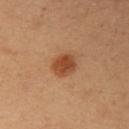Q: Was a biopsy performed?
A: total-body-photography surveillance lesion; no biopsy
Q: What kind of image is this?
A: ~15 mm crop, total-body skin-cancer survey
Q: What is the anatomic site?
A: the right upper arm
Q: Who is the patient?
A: female, aged around 35
Q: Automated lesion metrics?
A: a footprint of about 6.5 mm², an outline eccentricity of about 0.5 (0 = round, 1 = elongated), and two-axis asymmetry of about 0.15; a nevus-likeness score of about 100/100 and a lesion-detection confidence of about 100/100
Q: What lighting was used for the tile?
A: cross-polarized illumination